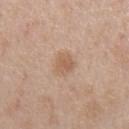Part of a total-body skin-imaging series; this lesion was reviewed on a skin check and was not flagged for biopsy. From the abdomen. Cropped from a whole-body photographic skin survey; the tile spans about 15 mm. A male patient aged 53 to 57. The recorded lesion diameter is about 2.5 mm.